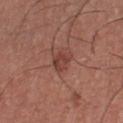Assessment: No biopsy was performed on this lesion — it was imaged during a full skin examination and was not determined to be concerning. Background: Imaged with white-light lighting. Located on the chest. A male subject, in their mid-30s. A region of skin cropped from a whole-body photographic capture, roughly 15 mm wide. The total-body-photography lesion software estimated a footprint of about 4.5 mm² and a symmetry-axis asymmetry near 0.3. And it measured a mean CIELAB color near L≈42 a*≈24 b*≈25, a lesion–skin lightness drop of about 8, and a normalized lesion–skin contrast near 7. The analysis additionally found border irregularity of about 2.5 on a 0–10 scale, a color-variation rating of about 2.5/10, and a peripheral color-asymmetry measure near 1. About 2.5 mm across.